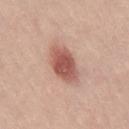subject: female, aged 18 to 22 | site: the leg | imaging modality: 15 mm crop, total-body photography.